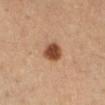Captured during whole-body skin photography for melanoma surveillance; the lesion was not biopsied. The lesion-visualizer software estimated a shape eccentricity near 0.55 and a symmetry-axis asymmetry near 0.15. It also reported roughly 15 lightness units darker than nearby skin and a lesion-to-skin contrast of about 11 (normalized; higher = more distinct). It also reported internal color variation of about 3.5 on a 0–10 scale. A lesion tile, about 15 mm wide, cut from a 3D total-body photograph. The subject is a female aged 28–32. The lesion is located on the right lower leg. Imaged with cross-polarized lighting.